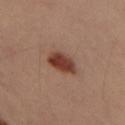A male patient aged 38 to 42. Located on the left thigh. A 15 mm crop from a total-body photograph taken for skin-cancer surveillance. Imaged with cross-polarized lighting.